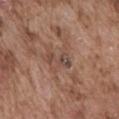* follow-up — no biopsy performed (imaged during a skin exam)
* subject — male, about 75 years old
* automated metrics — a lesion color around L≈46 a*≈17 b*≈24 in CIELAB and about 8 CIELAB-L* units darker than the surrounding skin; border irregularity of about 5.5 on a 0–10 scale, a color-variation rating of about 4/10, and radial color variation of about 1.5; a nevus-likeness score of about 0/100
* location — the abdomen
* image source — 15 mm crop, total-body photography
* lesion size — ≈3.5 mm